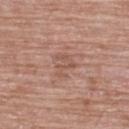Assessment: This lesion was catalogued during total-body skin photography and was not selected for biopsy. Background: A 15 mm close-up extracted from a 3D total-body photography capture. Automated image analysis of the tile measured a footprint of about 4.5 mm², a shape eccentricity near 0.7, and two-axis asymmetry of about 0.55. It also reported internal color variation of about 1 on a 0–10 scale and a peripheral color-asymmetry measure near 0.5. And it measured a nevus-likeness score of about 0/100 and a lesion-detection confidence of about 100/100. The lesion is on the upper back. A female subject aged around 60. The recorded lesion diameter is about 3 mm.